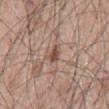follow-up: no biopsy performed (imaged during a skin exam) | automated metrics: a footprint of about 3 mm², a shape eccentricity near 0.8, and two-axis asymmetry of about 0.4; a lesion color around L≈49 a*≈18 b*≈25 in CIELAB, about 11 CIELAB-L* units darker than the surrounding skin, and a normalized lesion–skin contrast near 8.5 | subject: male, in their mid- to late 40s | lesion diameter: ~2.5 mm (longest diameter) | imaging modality: ~15 mm tile from a whole-body skin photo | illumination: white-light | location: the back.